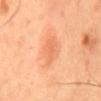{
  "biopsy_status": "not biopsied; imaged during a skin examination",
  "patient": {
    "sex": "male",
    "age_approx": 65
  },
  "image": {
    "source": "total-body photography crop",
    "field_of_view_mm": 15
  },
  "site": "back"
}